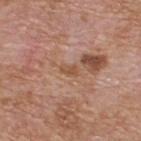Findings:
* biopsy status: total-body-photography surveillance lesion; no biopsy
* tile lighting: white-light illumination
* patient: male, aged around 60
* size: about 2.5 mm
* imaging modality: ~15 mm crop, total-body skin-cancer survey
* anatomic site: the upper back